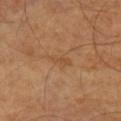Impression:
The lesion was photographed on a routine skin check and not biopsied; there is no pathology result.
Context:
This is a cross-polarized tile. A male patient, in their mid- to late 60s. A 15 mm close-up extracted from a 3D total-body photography capture. About 3 mm across.